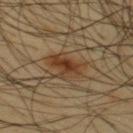Case summary:
* notes — imaged on a skin check; not biopsied
* image — ~15 mm tile from a whole-body skin photo
* size — ≈4 mm
* body site — the upper back
* subject — male, about 45 years old
* image-analysis metrics — a nevus-likeness score of about 95/100 and a lesion-detection confidence of about 100/100